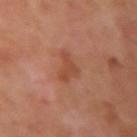follow-up: imaged on a skin check; not biopsied | anatomic site: the left arm | patient: male, aged 48 to 52 | illumination: cross-polarized | image source: ~15 mm tile from a whole-body skin photo | size: about 3.5 mm | image-analysis metrics: a lesion area of about 4.5 mm², a shape eccentricity near 0.75, and a shape-asymmetry score of about 0.65 (0 = symmetric).